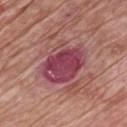– follow-up: catalogued during a skin exam; not biopsied
– subject: male, approximately 65 years of age
– image: 15 mm crop, total-body photography
– tile lighting: white-light
– lesion diameter: about 6 mm
– location: the chest